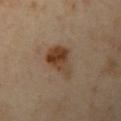Impression: Recorded during total-body skin imaging; not selected for excision or biopsy. Background: A 15 mm close-up extracted from a 3D total-body photography capture. A female patient in their 40s. The lesion-visualizer software estimated an area of roughly 10 mm², an outline eccentricity of about 0.75 (0 = round, 1 = elongated), and two-axis asymmetry of about 0.35. And it measured an average lesion color of about L≈41 a*≈17 b*≈31 (CIELAB), a lesion–skin lightness drop of about 11, and a lesion-to-skin contrast of about 9.5 (normalized; higher = more distinct). The tile uses cross-polarized illumination. Longest diameter approximately 4.5 mm. The lesion is on the left upper arm.